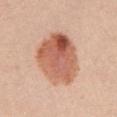Clinical impression: This lesion was catalogued during total-body skin photography and was not selected for biopsy. Acquisition and patient details: A female subject in their mid-20s. The lesion is located on the left upper arm. Automated tile analysis of the lesion measured a lesion area of about 31 mm², an outline eccentricity of about 0.6 (0 = round, 1 = elongated), and two-axis asymmetry of about 0.15. The analysis additionally found a lesion color around L≈60 a*≈25 b*≈32 in CIELAB, about 14 CIELAB-L* units darker than the surrounding skin, and a lesion-to-skin contrast of about 9 (normalized; higher = more distinct). The analysis additionally found a border-irregularity index near 1.5/10, a color-variation rating of about 9.5/10, and peripheral color asymmetry of about 3.5. The analysis additionally found a nevus-likeness score of about 100/100 and a detector confidence of about 100 out of 100 that the crop contains a lesion. Approximately 7 mm at its widest. The tile uses white-light illumination. Cropped from a whole-body photographic skin survey; the tile spans about 15 mm.